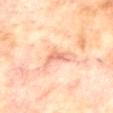| feature | finding |
|---|---|
| workup | imaged on a skin check; not biopsied |
| image-analysis metrics | a within-lesion color-variation index near 2/10 and a peripheral color-asymmetry measure near 0.5; a classifier nevus-likeness of about 0/100 and lesion-presence confidence of about 90/100 |
| illumination | cross-polarized |
| acquisition | ~15 mm crop, total-body skin-cancer survey |
| anatomic site | the mid back |
| subject | male, aged around 70 |
| lesion diameter | ~2.5 mm (longest diameter) |